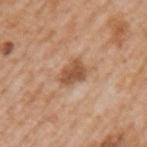A male subject, aged around 65.
A lesion tile, about 15 mm wide, cut from a 3D total-body photograph.
The lesion is on the left upper arm.
The recorded lesion diameter is about 3.5 mm.
Imaged with white-light lighting.